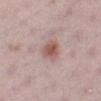Q: Is there a histopathology result?
A: total-body-photography surveillance lesion; no biopsy
Q: Lesion location?
A: the leg
Q: Patient demographics?
A: female, aged approximately 30
Q: Illumination type?
A: white-light illumination
Q: What is the imaging modality?
A: total-body-photography crop, ~15 mm field of view
Q: Lesion size?
A: about 3 mm